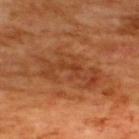Q: What did automated image analysis measure?
A: a mean CIELAB color near L≈42 a*≈26 b*≈36 and roughly 8 lightness units darker than nearby skin; a border-irregularity index near 8/10, a within-lesion color-variation index near 4.5/10, and peripheral color asymmetry of about 1.5; a nevus-likeness score of about 45/100 and a detector confidence of about 95 out of 100 that the crop contains a lesion
Q: Illumination type?
A: cross-polarized illumination
Q: Who is the patient?
A: male, aged approximately 65
Q: How large is the lesion?
A: ≈7.5 mm
Q: Lesion location?
A: the upper back
Q: What kind of image is this?
A: 15 mm crop, total-body photography